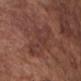Impression:
Part of a total-body skin-imaging series; this lesion was reviewed on a skin check and was not flagged for biopsy.
Acquisition and patient details:
A 15 mm crop from a total-body photograph taken for skin-cancer surveillance. An algorithmic analysis of the crop reported a footprint of about 15 mm², an outline eccentricity of about 0.95 (0 = round, 1 = elongated), and two-axis asymmetry of about 0.3. The tile uses white-light illumination. From the chest. A male patient about 75 years old.